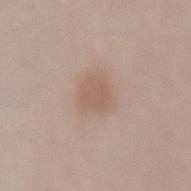  biopsy_status: not biopsied; imaged during a skin examination
  lesion_size:
    long_diameter_mm_approx: 3.0
  patient:
    sex: female
    age_approx: 30
  site: lower back
  image:
    source: total-body photography crop
    field_of_view_mm: 15
  automated_metrics:
    eccentricity: 0.55
    shape_asymmetry: 0.2
    cielab_L: 58
    cielab_a: 16
    cielab_b: 26
    vs_skin_darker_L: 7.0
    vs_skin_contrast_norm: 5.5
    border_irregularity_0_10: 1.5
    color_variation_0_10: 1.5
    peripheral_color_asymmetry: 0.5
    nevus_likeness_0_100: 50
    lesion_detection_confidence_0_100: 100
  lighting: white-light